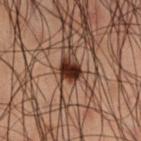Impression: The lesion was tiled from a total-body skin photograph and was not biopsied. Background: A 15 mm close-up extracted from a 3D total-body photography capture. Longest diameter approximately 2.5 mm. A male subject, about 50 years old. On the right thigh.